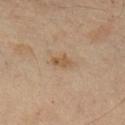The lesion was tiled from a total-body skin photograph and was not biopsied. A male patient, aged approximately 45. The tile uses cross-polarized illumination. Longest diameter approximately 2.5 mm. On the arm. A lesion tile, about 15 mm wide, cut from a 3D total-body photograph.